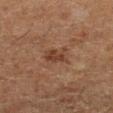Located on the left lower leg. A female patient aged 48 to 52. The tile uses cross-polarized illumination. Automated tile analysis of the lesion measured a lesion area of about 5.5 mm², a shape eccentricity near 0.7, and a symmetry-axis asymmetry near 0.35. A close-up tile cropped from a whole-body skin photograph, about 15 mm across.